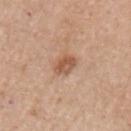No biopsy was performed on this lesion — it was imaged during a full skin examination and was not determined to be concerning.
A close-up tile cropped from a whole-body skin photograph, about 15 mm across.
The tile uses white-light illumination.
From the left upper arm.
A female patient, aged approximately 60.